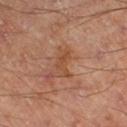Q: Is there a histopathology result?
A: imaged on a skin check; not biopsied
Q: What are the patient's age and sex?
A: male, in their 70s
Q: What did automated image analysis measure?
A: an area of roughly 3.5 mm², a shape eccentricity near 0.9, and a symmetry-axis asymmetry near 0.6; a lesion–skin lightness drop of about 7 and a normalized lesion–skin contrast near 6.5
Q: How large is the lesion?
A: ≈3 mm
Q: What lighting was used for the tile?
A: cross-polarized illumination
Q: What kind of image is this?
A: ~15 mm crop, total-body skin-cancer survey
Q: Where on the body is the lesion?
A: the left lower leg